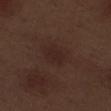follow-up: total-body-photography surveillance lesion; no biopsy
image: total-body-photography crop, ~15 mm field of view
illumination: white-light
location: the right thigh
patient: male, approximately 70 years of age
lesion size: about 2.5 mm